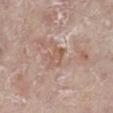Q: Was this lesion biopsied?
A: no biopsy performed (imaged during a skin exam)
Q: What did automated image analysis measure?
A: an eccentricity of roughly 0.7 and two-axis asymmetry of about 0.4; a lesion–skin lightness drop of about 7 and a normalized border contrast of about 5.5; a classifier nevus-likeness of about 0/100 and a detector confidence of about 85 out of 100 that the crop contains a lesion
Q: Who is the patient?
A: female, aged 68 to 72
Q: What is the anatomic site?
A: the left lower leg
Q: How large is the lesion?
A: ~2.5 mm (longest diameter)
Q: How was this image acquired?
A: ~15 mm tile from a whole-body skin photo